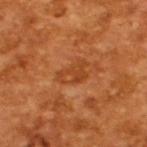tile lighting: cross-polarized
patient: male, in their mid- to late 60s
image-analysis metrics: an eccentricity of roughly 0.8 and a symmetry-axis asymmetry near 0.45; an average lesion color of about L≈43 a*≈28 b*≈41 (CIELAB) and a lesion-to-skin contrast of about 5.5 (normalized; higher = more distinct)
image: total-body-photography crop, ~15 mm field of view
lesion size: ~3.5 mm (longest diameter)